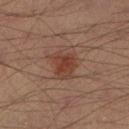  biopsy_status: not biopsied; imaged during a skin examination
  lesion_size:
    long_diameter_mm_approx: 3.5
  patient:
    sex: male
    age_approx: 40
  automated_metrics:
    nevus_likeness_0_100: 75
  lighting: cross-polarized
  image:
    source: total-body photography crop
    field_of_view_mm: 15
  site: left lower leg